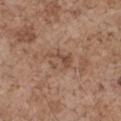follow-up: no biopsy performed (imaged during a skin exam)
patient: male, in their 70s
body site: the arm
image source: ~15 mm crop, total-body skin-cancer survey
lesion size: ≈3.5 mm
tile lighting: white-light
automated metrics: an eccentricity of roughly 0.6 and a shape-asymmetry score of about 0.25 (0 = symmetric); a lesion color around L≈50 a*≈18 b*≈28 in CIELAB, a lesion–skin lightness drop of about 7, and a normalized border contrast of about 5; a border-irregularity rating of about 3/10